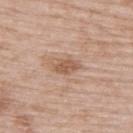Q: Is there a histopathology result?
A: no biopsy performed (imaged during a skin exam)